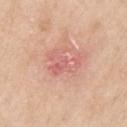Assessment: The lesion was tiled from a total-body skin photograph and was not biopsied. Context: Located on the left upper arm. The patient is a female aged around 70. A close-up tile cropped from a whole-body skin photograph, about 15 mm across. Captured under white-light illumination. About 4 mm across.